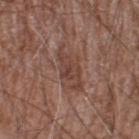The lesion was photographed on a routine skin check and not biopsied; there is no pathology result. The patient is a male aged around 60. The lesion is located on the left thigh. A roughly 15 mm field-of-view crop from a total-body skin photograph.